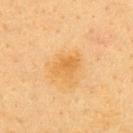Recorded during total-body skin imaging; not selected for excision or biopsy.
Measured at roughly 3.5 mm in maximum diameter.
A close-up tile cropped from a whole-body skin photograph, about 15 mm across.
From the back.
The subject is a female in their 40s.